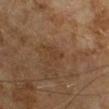biopsy status: no biopsy performed (imaged during a skin exam)
subject: male, aged 63–67
lesion size: ≈2.5 mm
imaging modality: ~15 mm tile from a whole-body skin photo